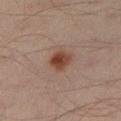  biopsy_status: not biopsied; imaged during a skin examination
  site: left lower leg
  image:
    source: total-body photography crop
    field_of_view_mm: 15
  patient:
    sex: male
    age_approx: 45